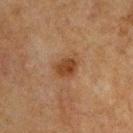Recorded during total-body skin imaging; not selected for excision or biopsy.
The tile uses cross-polarized illumination.
The lesion-visualizer software estimated a lesion area of about 6.5 mm², an eccentricity of roughly 0.7, and a shape-asymmetry score of about 0.2 (0 = symmetric). And it measured a lesion color around L≈35 a*≈18 b*≈29 in CIELAB, about 8 CIELAB-L* units darker than the surrounding skin, and a normalized lesion–skin contrast near 8. It also reported radial color variation of about 1.
A 15 mm close-up extracted from a 3D total-body photography capture.
A male subject, about 75 years old.
The recorded lesion diameter is about 3.5 mm.
Located on the chest.